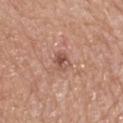Q: Was a biopsy performed?
A: catalogued during a skin exam; not biopsied
Q: Lesion location?
A: the back
Q: What kind of image is this?
A: ~15 mm crop, total-body skin-cancer survey
Q: Lesion size?
A: ~2.5 mm (longest diameter)
Q: Who is the patient?
A: male, aged approximately 75
Q: How was the tile lit?
A: white-light